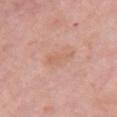Recorded during total-body skin imaging; not selected for excision or biopsy. From the chest. The tile uses white-light illumination. A lesion tile, about 15 mm wide, cut from a 3D total-body photograph. A female subject, in their mid-60s.